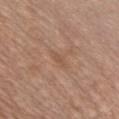No biopsy was performed on this lesion — it was imaged during a full skin examination and was not determined to be concerning.
The subject is a male aged 38–42.
A 15 mm crop from a total-body photograph taken for skin-cancer surveillance.
The lesion is located on the front of the torso.
Captured under white-light illumination.